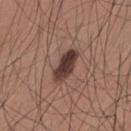Recorded during total-body skin imaging; not selected for excision or biopsy. A male subject, roughly 35 years of age. The recorded lesion diameter is about 4.5 mm. The lesion-visualizer software estimated a shape eccentricity near 0.85 and a symmetry-axis asymmetry near 0.2. The software also gave a mean CIELAB color near L≈38 a*≈18 b*≈21, roughly 15 lightness units darker than nearby skin, and a normalized border contrast of about 12.5. It also reported border irregularity of about 2.5 on a 0–10 scale, a within-lesion color-variation index near 5/10, and peripheral color asymmetry of about 2. The lesion is on the mid back. A 15 mm crop from a total-body photograph taken for skin-cancer surveillance.